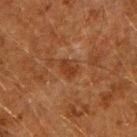biopsy_status: not biopsied; imaged during a skin examination
lesion_size:
  long_diameter_mm_approx: 2.5
patient:
  sex: male
  age_approx: 60
site: right upper arm
lighting: cross-polarized
image:
  source: total-body photography crop
  field_of_view_mm: 15